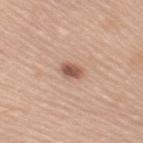workup — total-body-photography surveillance lesion; no biopsy
anatomic site — the right upper arm
illumination — white-light
image source — ~15 mm crop, total-body skin-cancer survey
lesion diameter — ~2.5 mm (longest diameter)
patient — female, aged around 60